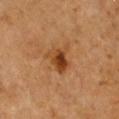Captured during whole-body skin photography for melanoma surveillance; the lesion was not biopsied. The lesion is located on the chest. A female subject aged 63–67. A region of skin cropped from a whole-body photographic capture, roughly 15 mm wide.